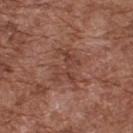Clinical impression: Recorded during total-body skin imaging; not selected for excision or biopsy. Context: From the back. The patient is a male roughly 75 years of age. Automated tile analysis of the lesion measured a lesion area of about 8.5 mm², an eccentricity of roughly 0.6, and two-axis asymmetry of about 0.5. The tile uses white-light illumination. Longest diameter approximately 4 mm. A 15 mm close-up tile from a total-body photography series done for melanoma screening.